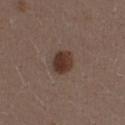• follow-up: catalogued during a skin exam; not biopsied
• lighting: white-light illumination
• image: ~15 mm crop, total-body skin-cancer survey
• anatomic site: the right thigh
• patient: female, roughly 30 years of age